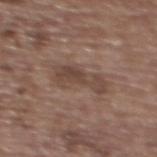<case>
<biopsy_status>not biopsied; imaged during a skin examination</biopsy_status>
<automated_metrics>
  <area_mm2_approx>8.5</area_mm2_approx>
  <eccentricity>0.9</eccentricity>
  <shape_asymmetry>0.45</shape_asymmetry>
  <cielab_L>43</cielab_L>
  <cielab_a>16</cielab_a>
  <cielab_b>23</cielab_b>
  <vs_skin_contrast_norm>6.5</vs_skin_contrast_norm>
</automated_metrics>
<patient>
  <sex>female</sex>
  <age_approx>65</age_approx>
</patient>
<lesion_size>
  <long_diameter_mm_approx>5.0</long_diameter_mm_approx>
</lesion_size>
<lighting>white-light</lighting>
<image>
  <source>total-body photography crop</source>
  <field_of_view_mm>15</field_of_view_mm>
</image>
<site>upper back</site>
</case>